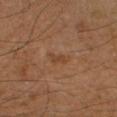notes = no biopsy performed (imaged during a skin exam)
subject = male, in their mid-50s
TBP lesion metrics = an automated nevus-likeness rating near 0 out of 100 and a lesion-detection confidence of about 100/100
imaging modality = ~15 mm tile from a whole-body skin photo
location = the right forearm